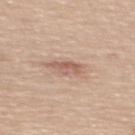<lesion>
  <biopsy_status>not biopsied; imaged during a skin examination</biopsy_status>
  <patient>
    <sex>female</sex>
    <age_approx>55</age_approx>
  </patient>
  <image>
    <source>total-body photography crop</source>
    <field_of_view_mm>15</field_of_view_mm>
  </image>
  <lesion_size>
    <long_diameter_mm_approx>3.0</long_diameter_mm_approx>
  </lesion_size>
  <site>upper back</site>
</lesion>